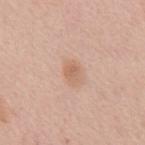  biopsy_status: not biopsied; imaged during a skin examination
  automated_metrics:
    cielab_L: 63
    cielab_a: 20
    cielab_b: 31
    vs_skin_contrast_norm: 6.0
    nevus_likeness_0_100: 35
    lesion_detection_confidence_0_100: 100
  patient:
    sex: male
    age_approx: 55
  lighting: white-light
  site: chest
  lesion_size:
    long_diameter_mm_approx: 2.5
  image:
    source: total-body photography crop
    field_of_view_mm: 15